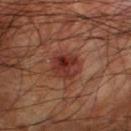Impression:
Captured during whole-body skin photography for melanoma surveillance; the lesion was not biopsied.
Clinical summary:
Cropped from a whole-body photographic skin survey; the tile spans about 15 mm. The patient is a male roughly 60 years of age. From the right lower leg.